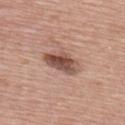The lesion was photographed on a routine skin check and not biopsied; there is no pathology result. A 15 mm close-up extracted from a 3D total-body photography capture. Located on the chest. A female subject, approximately 65 years of age.